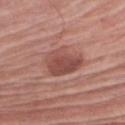biopsy status = no biopsy performed (imaged during a skin exam); body site = the right upper arm; illumination = white-light illumination; subject = male, aged 78 to 82; image source = ~15 mm crop, total-body skin-cancer survey.